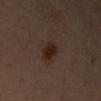Recorded during total-body skin imaging; not selected for excision or biopsy.
The subject is a female about 55 years old.
Located on the left arm.
Cropped from a total-body skin-imaging series; the visible field is about 15 mm.
This is a cross-polarized tile.